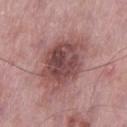Impression: Part of a total-body skin-imaging series; this lesion was reviewed on a skin check and was not flagged for biopsy. Clinical summary: This image is a 15 mm lesion crop taken from a total-body photograph. The patient is a male aged 53–57. Measured at roughly 7 mm in maximum diameter. This is a white-light tile. The total-body-photography lesion software estimated a border-irregularity index near 2.5/10 and internal color variation of about 6.5 on a 0–10 scale. The software also gave a classifier nevus-likeness of about 20/100. From the left thigh.